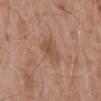Notes:
• imaging modality · ~15 mm crop, total-body skin-cancer survey
• site · the mid back
• patient · male, aged around 75